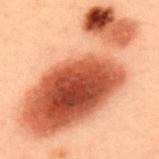Findings:
- notes — catalogued during a skin exam; not biopsied
- site — the upper back
- patient — male, aged approximately 55
- imaging modality — total-body-photography crop, ~15 mm field of view
- size — ~15 mm (longest diameter)